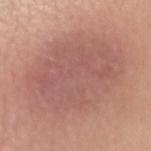<case>
<biopsy_status>not biopsied; imaged during a skin examination</biopsy_status>
<patient>
  <sex>female</sex>
  <age_approx>45</age_approx>
</patient>
<image>
  <source>total-body photography crop</source>
  <field_of_view_mm>15</field_of_view_mm>
</image>
<site>right upper arm</site>
</case>